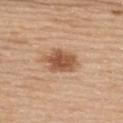Q: Who is the patient?
A: female, approximately 65 years of age
Q: What is the anatomic site?
A: the upper back
Q: What is the imaging modality?
A: total-body-photography crop, ~15 mm field of view
Q: What lighting was used for the tile?
A: white-light illumination